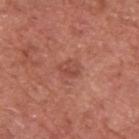This is a white-light tile.
The subject is a male aged approximately 75.
Approximately 2.5 mm at its widest.
The lesion-visualizer software estimated a lesion–skin lightness drop of about 7. And it measured a lesion-detection confidence of about 100/100.
From the upper back.
Cropped from a total-body skin-imaging series; the visible field is about 15 mm.